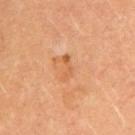workup = imaged on a skin check; not biopsied | image source = ~15 mm tile from a whole-body skin photo | subject = female, in their mid-30s | site = the right upper arm.